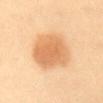<record>
<biopsy_status>not biopsied; imaged during a skin examination</biopsy_status>
<lesion_size>
  <long_diameter_mm_approx>5.5</long_diameter_mm_approx>
</lesion_size>
<lighting>cross-polarized</lighting>
<image>
  <source>total-body photography crop</source>
  <field_of_view_mm>15</field_of_view_mm>
</image>
<patient>
  <sex>female</sex>
  <age_approx>50</age_approx>
</patient>
<site>abdomen</site>
</record>